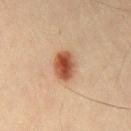follow-up = imaged on a skin check; not biopsied | image source = 15 mm crop, total-body photography | illumination = cross-polarized illumination | size = ≈3.5 mm | anatomic site = the front of the torso | image-analysis metrics = a mean CIELAB color near L≈44 a*≈21 b*≈30, roughly 13 lightness units darker than nearby skin, and a normalized border contrast of about 10.5 | subject = male, aged approximately 75.